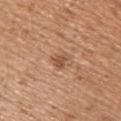lesion size = ≈2.5 mm; imaging modality = total-body-photography crop, ~15 mm field of view; illumination = white-light illumination; site = the upper back; patient = female, approximately 45 years of age.